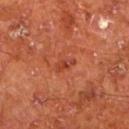Case summary:
* biopsy status · imaged on a skin check; not biopsied
* image · 15 mm crop, total-body photography
* automated lesion analysis · a footprint of about 3 mm², an eccentricity of roughly 0.85, and a shape-asymmetry score of about 0.35 (0 = symmetric); a mean CIELAB color near L≈42 a*≈32 b*≈34, a lesion–skin lightness drop of about 7, and a normalized border contrast of about 6; an automated nevus-likeness rating near 0 out of 100 and a lesion-detection confidence of about 100/100
* lesion diameter · about 2.5 mm
* site · the leg
* patient · male, in their mid- to late 60s